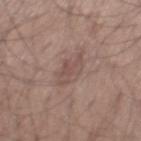The lesion was tiled from a total-body skin photograph and was not biopsied. Automated tile analysis of the lesion measured an area of roughly 7 mm², a shape eccentricity near 0.75, and a symmetry-axis asymmetry near 0.25. It also reported border irregularity of about 3 on a 0–10 scale and a within-lesion color-variation index near 2/10. The software also gave a nevus-likeness score of about 0/100 and a lesion-detection confidence of about 100/100. On the mid back. A male subject, aged approximately 35. A roughly 15 mm field-of-view crop from a total-body skin photograph. Longest diameter approximately 4 mm. Captured under white-light illumination.